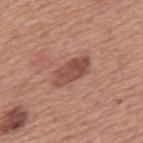Case summary:
* biopsy status: imaged on a skin check; not biopsied
* subject: male, aged approximately 75
* diameter: ≈4.5 mm
* location: the mid back
* acquisition: total-body-photography crop, ~15 mm field of view